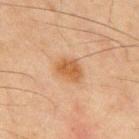Automated image analysis of the tile measured a lesion area of about 6.5 mm², an outline eccentricity of about 0.65 (0 = round, 1 = elongated), and a symmetry-axis asymmetry near 0.15. The software also gave a mean CIELAB color near L≈47 a*≈19 b*≈34, roughly 9 lightness units darker than nearby skin, and a normalized border contrast of about 8. The software also gave a classifier nevus-likeness of about 95/100 and lesion-presence confidence of about 100/100. Located on the mid back. This image is a 15 mm lesion crop taken from a total-body photograph. A male patient in their mid-40s. Longest diameter approximately 3.5 mm.